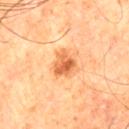Part of a total-body skin-imaging series; this lesion was reviewed on a skin check and was not flagged for biopsy. Cropped from a total-body skin-imaging series; the visible field is about 15 mm. The recorded lesion diameter is about 3.5 mm. On the right thigh. A male subject, aged 78–82.